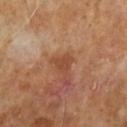Imaged during a routine full-body skin examination; the lesion was not biopsied and no histopathology is available. Cropped from a total-body skin-imaging series; the visible field is about 15 mm. Automated image analysis of the tile measured an average lesion color of about L≈45 a*≈23 b*≈32 (CIELAB) and a normalized lesion–skin contrast near 6. The software also gave internal color variation of about 1.5 on a 0–10 scale and peripheral color asymmetry of about 0.5. And it measured a classifier nevus-likeness of about 0/100. Longest diameter approximately 2.5 mm. A male subject, aged 63–67.